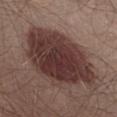workup: total-body-photography surveillance lesion; no biopsy
lesion size: ≈10.5 mm
illumination: white-light illumination
subject: male, in their mid- to late 50s
imaging modality: ~15 mm crop, total-body skin-cancer survey
site: the abdomen